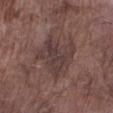Clinical impression: This lesion was catalogued during total-body skin photography and was not selected for biopsy. Context: A male subject in their mid- to late 70s. Imaged with white-light lighting. Cropped from a whole-body photographic skin survey; the tile spans about 15 mm. The lesion-visualizer software estimated an average lesion color of about L≈38 a*≈16 b*≈18 (CIELAB), a lesion–skin lightness drop of about 7, and a normalized border contrast of about 6.5. Measured at roughly 5.5 mm in maximum diameter. From the right lower leg.